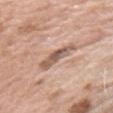Captured during whole-body skin photography for melanoma surveillance; the lesion was not biopsied. A female subject, aged 73 to 77. The tile uses white-light illumination. The lesion's longest dimension is about 4.5 mm. Located on the right upper arm. A lesion tile, about 15 mm wide, cut from a 3D total-body photograph. Automated image analysis of the tile measured a lesion area of about 7 mm² and an eccentricity of roughly 0.9. And it measured border irregularity of about 4 on a 0–10 scale, internal color variation of about 6.5 on a 0–10 scale, and radial color variation of about 2.